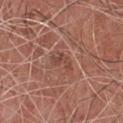Clinical impression: The lesion was tiled from a total-body skin photograph and was not biopsied. Acquisition and patient details: A male patient about 75 years old. From the chest. Measured at roughly 3 mm in maximum diameter. Cropped from a total-body skin-imaging series; the visible field is about 15 mm. This is a white-light tile.